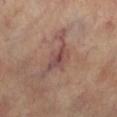image source: ~15 mm crop, total-body skin-cancer survey; site: the right lower leg; lesion size: ≈4 mm; tile lighting: cross-polarized; patient: female, about 65 years old; image-analysis metrics: peripheral color asymmetry of about 1.5.